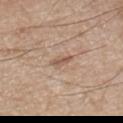Clinical impression: Imaged during a routine full-body skin examination; the lesion was not biopsied and no histopathology is available. Context: A male patient, in their mid-70s. From the chest. Cropped from a total-body skin-imaging series; the visible field is about 15 mm.